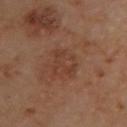Part of a total-body skin-imaging series; this lesion was reviewed on a skin check and was not flagged for biopsy. A 15 mm close-up tile from a total-body photography series done for melanoma screening. The subject is a male aged around 65. Longest diameter approximately 4 mm. Located on the back. This is a cross-polarized tile.